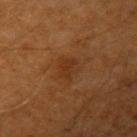Assessment:
Part of a total-body skin-imaging series; this lesion was reviewed on a skin check and was not flagged for biopsy.
Image and clinical context:
The lesion is on the left upper arm. The total-body-photography lesion software estimated an area of roughly 5.5 mm², an eccentricity of roughly 0.5, and a symmetry-axis asymmetry near 0.3. The software also gave a border-irregularity rating of about 3.5/10, a within-lesion color-variation index near 2.5/10, and peripheral color asymmetry of about 1. The software also gave a nevus-likeness score of about 0/100. Captured under cross-polarized illumination. Cropped from a whole-body photographic skin survey; the tile spans about 15 mm. About 3 mm across. The subject is a male roughly 60 years of age.